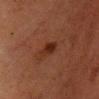biopsy status: catalogued during a skin exam; not biopsied
automated lesion analysis: an area of roughly 4.5 mm², an eccentricity of roughly 0.75, and a shape-asymmetry score of about 0.25 (0 = symmetric); an average lesion color of about L≈22 a*≈20 b*≈24 (CIELAB), roughly 7 lightness units darker than nearby skin, and a normalized lesion–skin contrast near 8; a border-irregularity rating of about 2.5/10, internal color variation of about 3 on a 0–10 scale, and a peripheral color-asymmetry measure near 1
patient: male, aged 48–52
image source: total-body-photography crop, ~15 mm field of view
site: the chest